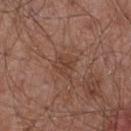follow-up: total-body-photography surveillance lesion; no biopsy
size: ~3 mm (longest diameter)
TBP lesion metrics: a lesion area of about 5.5 mm² and an outline eccentricity of about 0.35 (0 = round, 1 = elongated); peripheral color asymmetry of about 1; an automated nevus-likeness rating near 0 out of 100 and a detector confidence of about 100 out of 100 that the crop contains a lesion
tile lighting: white-light
anatomic site: the chest
patient: male, in their mid- to late 50s
image: total-body-photography crop, ~15 mm field of view